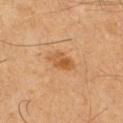Q: Is there a histopathology result?
A: no biopsy performed (imaged during a skin exam)
Q: Who is the patient?
A: male, aged 58 to 62
Q: Lesion location?
A: the right thigh
Q: What is the imaging modality?
A: ~15 mm crop, total-body skin-cancer survey